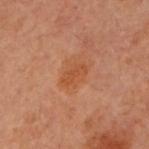<lesion>
<biopsy_status>not biopsied; imaged during a skin examination</biopsy_status>
</lesion>